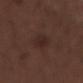follow-up=no biopsy performed (imaged during a skin exam); lesion size=~3 mm (longest diameter); site=the right forearm; tile lighting=white-light; subject=male, approximately 50 years of age; imaging modality=15 mm crop, total-body photography.